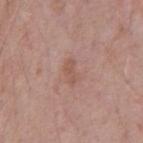Imaged during a routine full-body skin examination; the lesion was not biopsied and no histopathology is available. Longest diameter approximately 3 mm. A close-up tile cropped from a whole-body skin photograph, about 15 mm across. Automated tile analysis of the lesion measured an outline eccentricity of about 0.85 (0 = round, 1 = elongated) and a symmetry-axis asymmetry near 0.5. And it measured a border-irregularity index near 4.5/10. The software also gave a nevus-likeness score of about 0/100 and lesion-presence confidence of about 100/100. From the chest. A male patient roughly 70 years of age.